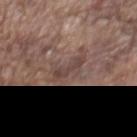workup = total-body-photography surveillance lesion; no biopsy | body site = the chest | patient = male, in their mid-70s | size = ~3 mm (longest diameter) | image source = 15 mm crop, total-body photography.